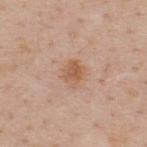Part of a total-body skin-imaging series; this lesion was reviewed on a skin check and was not flagged for biopsy. Approximately 3 mm at its widest. The tile uses white-light illumination. A lesion tile, about 15 mm wide, cut from a 3D total-body photograph. Automated image analysis of the tile measured a footprint of about 6 mm², an eccentricity of roughly 0.55, and a shape-asymmetry score of about 0.3 (0 = symmetric). A male subject aged around 40. From the upper back.